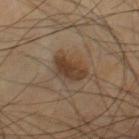Assessment:
Part of a total-body skin-imaging series; this lesion was reviewed on a skin check and was not flagged for biopsy.
Clinical summary:
Cropped from a whole-body photographic skin survey; the tile spans about 15 mm. A male subject about 70 years old. The lesion is located on the right thigh.